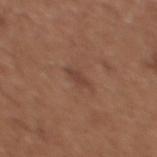follow-up: no biopsy performed (imaged during a skin exam) | location: the chest | illumination: white-light | acquisition: ~15 mm tile from a whole-body skin photo | automated lesion analysis: about 7 CIELAB-L* units darker than the surrounding skin and a normalized lesion–skin contrast near 6; border irregularity of about 3 on a 0–10 scale, internal color variation of about 0 on a 0–10 scale, and radial color variation of about 0; a detector confidence of about 100 out of 100 that the crop contains a lesion | subject: female, approximately 35 years of age.